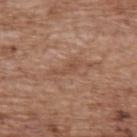notes: no biopsy performed (imaged during a skin exam).